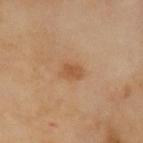Clinical impression: The lesion was photographed on a routine skin check and not biopsied; there is no pathology result. Image and clinical context: From the left upper arm. A close-up tile cropped from a whole-body skin photograph, about 15 mm across. The lesion's longest dimension is about 2.5 mm. A male patient, aged approximately 70. Captured under cross-polarized illumination.